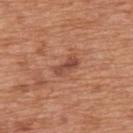Q: What is the anatomic site?
A: the back
Q: What lighting was used for the tile?
A: white-light
Q: What did automated image analysis measure?
A: an average lesion color of about L≈48 a*≈25 b*≈29 (CIELAB), a lesion–skin lightness drop of about 10, and a normalized lesion–skin contrast near 7.5; a border-irregularity index near 3/10, a color-variation rating of about 2/10, and a peripheral color-asymmetry measure near 0.5; a nevus-likeness score of about 25/100 and a detector confidence of about 100 out of 100 that the crop contains a lesion
Q: Who is the patient?
A: male, in their mid-60s
Q: How was this image acquired?
A: ~15 mm tile from a whole-body skin photo